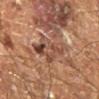| feature | finding |
|---|---|
| notes | catalogued during a skin exam; not biopsied |
| imaging modality | total-body-photography crop, ~15 mm field of view |
| subject | male, aged 58–62 |
| image-analysis metrics | about 10 CIELAB-L* units darker than the surrounding skin and a lesion-to-skin contrast of about 8 (normalized; higher = more distinct) |
| anatomic site | the right leg |
| illumination | cross-polarized illumination |
| lesion size | ≈6 mm |